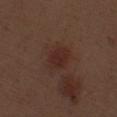Q: Is there a histopathology result?
A: total-body-photography surveillance lesion; no biopsy
Q: Lesion location?
A: the leg
Q: What are the patient's age and sex?
A: male, approximately 70 years of age
Q: How large is the lesion?
A: about 3.5 mm
Q: What kind of image is this?
A: total-body-photography crop, ~15 mm field of view
Q: Illumination type?
A: white-light illumination